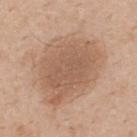workup — catalogued during a skin exam; not biopsied
site — the upper back
subject — male, aged approximately 60
automated lesion analysis — a footprint of about 40 mm², an eccentricity of roughly 0.65, and a shape-asymmetry score of about 0.2 (0 = symmetric); an average lesion color of about L≈58 a*≈18 b*≈30 (CIELAB) and a normalized lesion–skin contrast near 6.5
imaging modality — ~15 mm tile from a whole-body skin photo
lighting — white-light illumination